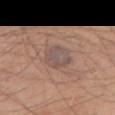<case>
  <biopsy_status>not biopsied; imaged during a skin examination</biopsy_status>
  <image>
    <source>total-body photography crop</source>
    <field_of_view_mm>15</field_of_view_mm>
  </image>
  <patient>
    <sex>male</sex>
    <age_approx>55</age_approx>
  </patient>
  <site>right upper arm</site>
  <lighting>white-light</lighting>
</case>